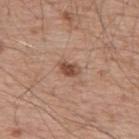  biopsy_status: not biopsied; imaged during a skin examination
  site: back
  image:
    source: total-body photography crop
    field_of_view_mm: 15
  patient:
    sex: male
    age_approx: 55